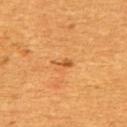workup: total-body-photography surveillance lesion; no biopsy
tile lighting: cross-polarized
TBP lesion metrics: an area of roughly 2.5 mm², a shape eccentricity near 0.85, and a symmetry-axis asymmetry near 0.45; a lesion–skin lightness drop of about 8 and a lesion-to-skin contrast of about 6 (normalized; higher = more distinct); a nevus-likeness score of about 10/100 and a detector confidence of about 100 out of 100 that the crop contains a lesion
subject: female, aged approximately 55
anatomic site: the back
image: ~15 mm tile from a whole-body skin photo